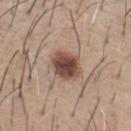This lesion was catalogued during total-body skin photography and was not selected for biopsy. Cropped from a whole-body photographic skin survey; the tile spans about 15 mm. Imaged with white-light lighting. The total-body-photography lesion software estimated a lesion color around L≈46 a*≈19 b*≈24 in CIELAB, about 16 CIELAB-L* units darker than the surrounding skin, and a normalized lesion–skin contrast near 11.5. The software also gave border irregularity of about 1.5 on a 0–10 scale, a within-lesion color-variation index near 5.5/10, and peripheral color asymmetry of about 1.5. The software also gave a classifier nevus-likeness of about 85/100 and a lesion-detection confidence of about 100/100. From the chest. The subject is a male in their 60s. Longest diameter approximately 3.5 mm.